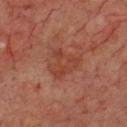Part of a total-body skin-imaging series; this lesion was reviewed on a skin check and was not flagged for biopsy. From the chest. The patient is a male in their 70s. A lesion tile, about 15 mm wide, cut from a 3D total-body photograph.